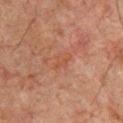  biopsy_status: not biopsied; imaged during a skin examination
  image:
    source: total-body photography crop
    field_of_view_mm: 15
  patient:
    sex: male
    age_approx: 60
  lesion_size:
    long_diameter_mm_approx: 2.5
  site: chest
  lighting: cross-polarized